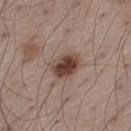{
  "biopsy_status": "not biopsied; imaged during a skin examination",
  "lesion_size": {
    "long_diameter_mm_approx": 3.5
  },
  "patient": {
    "sex": "male",
    "age_approx": 55
  },
  "lighting": "white-light",
  "site": "left thigh",
  "image": {
    "source": "total-body photography crop",
    "field_of_view_mm": 15
  },
  "automated_metrics": {
    "area_mm2_approx": 8.0,
    "shape_asymmetry": 0.2,
    "border_irregularity_0_10": 2.0,
    "nevus_likeness_0_100": 95
  }
}